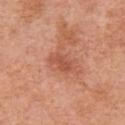follow-up: imaged on a skin check; not biopsied
image source: 15 mm crop, total-body photography
site: the chest
patient: male, roughly 70 years of age